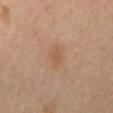Imaged during a routine full-body skin examination; the lesion was not biopsied and no histopathology is available.
Longest diameter approximately 3 mm.
Captured under cross-polarized illumination.
A roughly 15 mm field-of-view crop from a total-body skin photograph.
Located on the mid back.
A female patient, roughly 55 years of age.